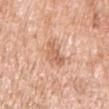biopsy status: total-body-photography surveillance lesion; no biopsy | subject: male, approximately 60 years of age | tile lighting: white-light illumination | body site: the left upper arm | image source: ~15 mm tile from a whole-body skin photo | lesion size: about 3.5 mm.